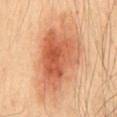<case>
<biopsy_status>not biopsied; imaged during a skin examination</biopsy_status>
<patient>
  <sex>male</sex>
  <age_approx>50</age_approx>
</patient>
<site>back</site>
<lesion_size>
  <long_diameter_mm_approx>10.0</long_diameter_mm_approx>
</lesion_size>
<image>
  <source>total-body photography crop</source>
  <field_of_view_mm>15</field_of_view_mm>
</image>
</case>